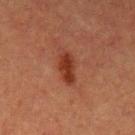Acquisition and patient details: The lesion is on the arm. A lesion tile, about 15 mm wide, cut from a 3D total-body photograph. Captured under cross-polarized illumination. The recorded lesion diameter is about 4 mm. A female subject, approximately 55 years of age.